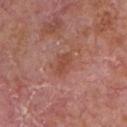Q: Was a biopsy performed?
A: total-body-photography surveillance lesion; no biopsy
Q: How large is the lesion?
A: ≈2.5 mm
Q: What is the imaging modality?
A: 15 mm crop, total-body photography
Q: What is the anatomic site?
A: the chest
Q: What are the patient's age and sex?
A: male, about 65 years old
Q: What lighting was used for the tile?
A: white-light